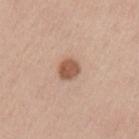  biopsy_status: not biopsied; imaged during a skin examination
  lesion_size:
    long_diameter_mm_approx: 2.5
  site: arm
  patient:
    sex: male
    age_approx: 40
  lighting: white-light
  image:
    source: total-body photography crop
    field_of_view_mm: 15
  automated_metrics:
    area_mm2_approx: 5.0
    eccentricity: 0.55
    cielab_L: 55
    cielab_a: 21
    cielab_b: 30
    vs_skin_darker_L: 14.0